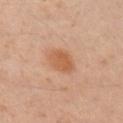biopsy_status: not biopsied; imaged during a skin examination
image:
  source: total-body photography crop
  field_of_view_mm: 15
site: right upper arm
patient:
  sex: male
  age_approx: 45